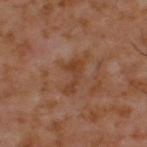Assessment: No biopsy was performed on this lesion — it was imaged during a full skin examination and was not determined to be concerning. Background: A lesion tile, about 15 mm wide, cut from a 3D total-body photograph. Imaged with cross-polarized lighting. Approximately 5 mm at its widest. A male subject about 60 years old. Located on the back.